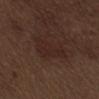The lesion was photographed on a routine skin check and not biopsied; there is no pathology result.
The lesion is located on the abdomen.
A 15 mm close-up extracted from a 3D total-body photography capture.
Imaged with white-light lighting.
A male patient, aged 68 to 72.